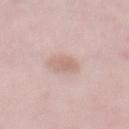Automated tile analysis of the lesion measured a lesion color around L≈65 a*≈18 b*≈25 in CIELAB, roughly 8 lightness units darker than nearby skin, and a normalized border contrast of about 5.5. The software also gave a peripheral color-asymmetry measure near 0.5. The software also gave an automated nevus-likeness rating near 55 out of 100 and lesion-presence confidence of about 100/100. About 3 mm across. Imaged with white-light lighting. A lesion tile, about 15 mm wide, cut from a 3D total-body photograph. A female subject roughly 50 years of age. The lesion is on the mid back.